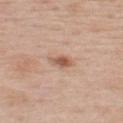Clinical impression:
No biopsy was performed on this lesion — it was imaged during a full skin examination and was not determined to be concerning.
Clinical summary:
A roughly 15 mm field-of-view crop from a total-body skin photograph. The lesion-visualizer software estimated an eccentricity of roughly 0.7 and a symmetry-axis asymmetry near 0.25. The lesion is on the back. This is a white-light tile. The subject is a male about 60 years old. About 2.5 mm across.